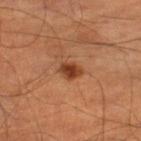follow-up: imaged on a skin check; not biopsied.